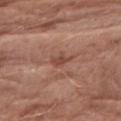{
  "biopsy_status": "not biopsied; imaged during a skin examination",
  "patient": {
    "sex": "female",
    "age_approx": "85+"
  },
  "image": {
    "source": "total-body photography crop",
    "field_of_view_mm": 15
  },
  "lesion_size": {
    "long_diameter_mm_approx": 2.5
  },
  "site": "right upper arm",
  "lighting": "white-light"
}